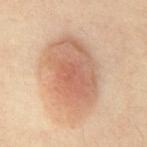From the abdomen. A 15 mm close-up tile from a total-body photography series done for melanoma screening. A male subject approximately 55 years of age. Approximately 8.5 mm at its widest. Captured under cross-polarized illumination.